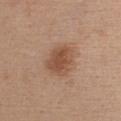workup = no biopsy performed (imaged during a skin exam); body site = the upper back; acquisition = ~15 mm crop, total-body skin-cancer survey; patient = female, roughly 45 years of age.